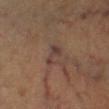Case summary:
* workup · catalogued during a skin exam; not biopsied
* subject · female, aged 63 to 67
* diameter · ~3 mm (longest diameter)
* image-analysis metrics · a border-irregularity index near 5/10, internal color variation of about 2 on a 0–10 scale, and peripheral color asymmetry of about 0.5
* location · the right lower leg
* acquisition · ~15 mm crop, total-body skin-cancer survey
* illumination · cross-polarized illumination